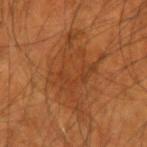The lesion was photographed on a routine skin check and not biopsied; there is no pathology result. The lesion is located on the left forearm. Cropped from a whole-body photographic skin survey; the tile spans about 15 mm. A male patient, in their mid-50s. Imaged with cross-polarized lighting. Approximately 9.5 mm at its widest.